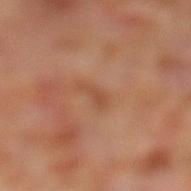Imaged during a routine full-body skin examination; the lesion was not biopsied and no histopathology is available. Located on the left lower leg. This is a cross-polarized tile. Longest diameter approximately 3 mm. Cropped from a whole-body photographic skin survey; the tile spans about 15 mm. The subject is a male aged approximately 70. The total-body-photography lesion software estimated an automated nevus-likeness rating near 0 out of 100 and a lesion-detection confidence of about 100/100.